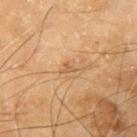The lesion was tiled from a total-body skin photograph and was not biopsied.
Cropped from a whole-body photographic skin survey; the tile spans about 15 mm.
A male patient, about 65 years old.
The lesion is on the arm.